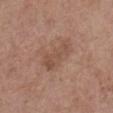Recorded during total-body skin imaging; not selected for excision or biopsy.
On the front of the torso.
The total-body-photography lesion software estimated an average lesion color of about L≈50 a*≈19 b*≈27 (CIELAB), roughly 7 lightness units darker than nearby skin, and a normalized lesion–skin contrast near 5.5. It also reported a color-variation rating of about 2.5/10. And it measured an automated nevus-likeness rating near 0 out of 100 and a detector confidence of about 100 out of 100 that the crop contains a lesion.
The subject is a female aged 63 to 67.
Longest diameter approximately 5 mm.
Cropped from a whole-body photographic skin survey; the tile spans about 15 mm.
This is a white-light tile.